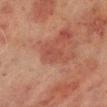| field | value |
|---|---|
| biopsy status | imaged on a skin check; not biopsied |
| acquisition | ~15 mm tile from a whole-body skin photo |
| patient | male, aged 68–72 |
| automated lesion analysis | a lesion area of about 3.5 mm² and an outline eccentricity of about 0.75 (0 = round, 1 = elongated); a border-irregularity rating of about 3.5/10, internal color variation of about 1 on a 0–10 scale, and peripheral color asymmetry of about 0.5 |
| tile lighting | cross-polarized illumination |
| anatomic site | the right lower leg |
| diameter | ≈2.5 mm |